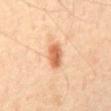workup = no biopsy performed (imaged during a skin exam)
TBP lesion metrics = a lesion color around L≈62 a*≈25 b*≈37 in CIELAB, roughly 13 lightness units darker than nearby skin, and a normalized lesion–skin contrast near 8.5; a border-irregularity index near 2/10, a within-lesion color-variation index near 3.5/10, and radial color variation of about 1
anatomic site = the abdomen
illumination = cross-polarized
patient = male, aged 43–47
acquisition = ~15 mm tile from a whole-body skin photo